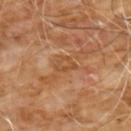Recorded during total-body skin imaging; not selected for excision or biopsy.
This image is a 15 mm lesion crop taken from a total-body photograph.
On the chest.
A male subject roughly 60 years of age.